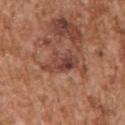{
  "site": "arm",
  "image": {
    "source": "total-body photography crop",
    "field_of_view_mm": 15
  },
  "patient": {
    "sex": "male",
    "age_approx": 45
  },
  "lighting": "white-light",
  "lesion_size": {
    "long_diameter_mm_approx": 5.0
  },
  "diagnosis": {
    "histopathology": "melanoma in situ",
    "malignancy": "malignant",
    "taxonomic_path": [
      "Malignant",
      "Malignant melanocytic proliferations (Melanoma)",
      "Melanoma in situ"
    ]
  }
}